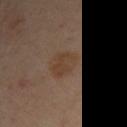Clinical summary:
A 15 mm close-up tile from a total-body photography series done for melanoma screening. A female patient, aged around 40. On the left arm.